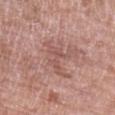Assessment:
Captured during whole-body skin photography for melanoma surveillance; the lesion was not biopsied.
Context:
The subject is a female roughly 70 years of age. The recorded lesion diameter is about 4 mm. Automated tile analysis of the lesion measured an average lesion color of about L≈53 a*≈22 b*≈25 (CIELAB), roughly 7 lightness units darker than nearby skin, and a normalized lesion–skin contrast near 4.5. The software also gave a classifier nevus-likeness of about 0/100 and lesion-presence confidence of about 90/100. A lesion tile, about 15 mm wide, cut from a 3D total-body photograph. The tile uses white-light illumination. Located on the left upper arm.